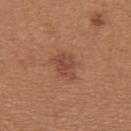  biopsy_status: not biopsied; imaged during a skin examination
  automated_metrics:
    cielab_L: 47
    cielab_a: 24
    cielab_b: 30
    vs_skin_contrast_norm: 6.5
  site: upper back
  image:
    source: total-body photography crop
    field_of_view_mm: 15
  patient:
    sex: female
    age_approx: 40
  lesion_size:
    long_diameter_mm_approx: 3.5